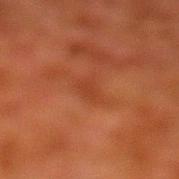The lesion was photographed on a routine skin check and not biopsied; there is no pathology result.
This is a cross-polarized tile.
A male subject in their 80s.
A close-up tile cropped from a whole-body skin photograph, about 15 mm across.
The total-body-photography lesion software estimated an outline eccentricity of about 0.85 (0 = round, 1 = elongated) and a symmetry-axis asymmetry near 0.35. The software also gave a border-irregularity rating of about 3.5/10, a within-lesion color-variation index near 1/10, and a peripheral color-asymmetry measure near 0.5. It also reported an automated nevus-likeness rating near 0 out of 100 and a lesion-detection confidence of about 100/100.
Approximately 2.5 mm at its widest.
From the left lower leg.